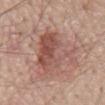Recorded during total-body skin imaging; not selected for excision or biopsy.
Captured under white-light illumination.
A close-up tile cropped from a whole-body skin photograph, about 15 mm across.
The subject is a male aged approximately 70.
The lesion's longest dimension is about 7 mm.
From the mid back.